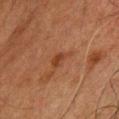follow-up = no biopsy performed (imaged during a skin exam)
imaging modality = 15 mm crop, total-body photography
body site = the chest
tile lighting = cross-polarized illumination
lesion diameter = about 3 mm
patient = male, roughly 60 years of age
TBP lesion metrics = a normalized border contrast of about 6.5; border irregularity of about 4.5 on a 0–10 scale and a peripheral color-asymmetry measure near 1; an automated nevus-likeness rating near 0 out of 100